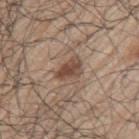A male patient aged approximately 70. Automated image analysis of the tile measured an average lesion color of about L≈47 a*≈17 b*≈26 (CIELAB), roughly 11 lightness units darker than nearby skin, and a normalized lesion–skin contrast near 8.5. It also reported a nevus-likeness score of about 75/100 and lesion-presence confidence of about 100/100. Captured under white-light illumination. A region of skin cropped from a whole-body photographic capture, roughly 15 mm wide. From the arm. The lesion's longest dimension is about 3.5 mm.